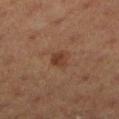No biopsy was performed on this lesion — it was imaged during a full skin examination and was not determined to be concerning.
The lesion-visualizer software estimated a footprint of about 4 mm² and an eccentricity of roughly 0.65. The software also gave a border-irregularity index near 2.5/10, internal color variation of about 2 on a 0–10 scale, and a peripheral color-asymmetry measure near 1. The software also gave a nevus-likeness score of about 20/100 and a lesion-detection confidence of about 100/100.
A female patient, aged 38 to 42.
On the left lower leg.
A 15 mm close-up extracted from a 3D total-body photography capture.